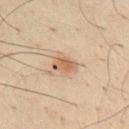The tile uses cross-polarized illumination. The lesion is on the chest. This image is a 15 mm lesion crop taken from a total-body photograph. The patient is a male aged 48 to 52. About 3 mm across.